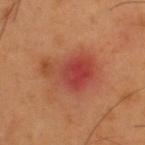A male patient, in their mid-50s. From the upper back. Approximately 6.5 mm at its widest. A 15 mm crop from a total-body photograph taken for skin-cancer surveillance.